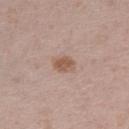<tbp_lesion>
  <biopsy_status>not biopsied; imaged during a skin examination</biopsy_status>
  <lesion_size>
    <long_diameter_mm_approx>2.5</long_diameter_mm_approx>
  </lesion_size>
  <site>left thigh</site>
  <patient>
    <sex>female</sex>
    <age_approx>30</age_approx>
  </patient>
  <image>
    <source>total-body photography crop</source>
    <field_of_view_mm>15</field_of_view_mm>
  </image>
</tbp_lesion>